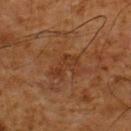Notes:
- follow-up · imaged on a skin check; not biopsied
- image source · ~15 mm tile from a whole-body skin photo
- subject · male, aged 58–62
- location · the upper back